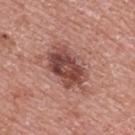| field | value |
|---|---|
| site | the back |
| imaging modality | ~15 mm tile from a whole-body skin photo |
| patient | male, roughly 70 years of age |
| tile lighting | white-light |